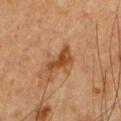Captured during whole-body skin photography for melanoma surveillance; the lesion was not biopsied.
A male patient, aged approximately 50.
A region of skin cropped from a whole-body photographic capture, roughly 15 mm wide.
On the chest.